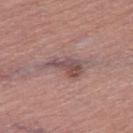<lesion>
  <biopsy_status>not biopsied; imaged during a skin examination</biopsy_status>
  <patient>
    <sex>female</sex>
    <age_approx>60</age_approx>
  </patient>
  <lesion_size>
    <long_diameter_mm_approx>5.5</long_diameter_mm_approx>
  </lesion_size>
  <site>right thigh</site>
  <image>
    <source>total-body photography crop</source>
    <field_of_view_mm>15</field_of_view_mm>
  </image>
  <automated_metrics>
    <area_mm2_approx>9.0</area_mm2_approx>
    <eccentricity>0.9</eccentricity>
    <shape_asymmetry>0.45</shape_asymmetry>
    <vs_skin_darker_L>10.0</vs_skin_darker_L>
    <nevus_likeness_0_100>5</nevus_likeness_0_100>
  </automated_metrics>
</lesion>